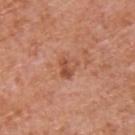<case>
  <site>upper back</site>
  <patient>
    <sex>male</sex>
    <age_approx>65</age_approx>
  </patient>
  <image>
    <source>total-body photography crop</source>
    <field_of_view_mm>15</field_of_view_mm>
  </image>
  <lighting>white-light</lighting>
  <automated_metrics>
    <area_mm2_approx>4.5</area_mm2_approx>
    <shape_asymmetry>0.4</shape_asymmetry>
    <cielab_L>52</cielab_L>
    <cielab_a>27</cielab_a>
    <cielab_b>33</cielab_b>
    <vs_skin_darker_L>9.0</vs_skin_darker_L>
    <vs_skin_contrast_norm>6.5</vs_skin_contrast_norm>
    <color_variation_0_10>6.0</color_variation_0_10>
    <peripheral_color_asymmetry>2.0</peripheral_color_asymmetry>
  </automated_metrics>
  <lesion_size>
    <long_diameter_mm_approx>2.5</long_diameter_mm_approx>
  </lesion_size>
</case>